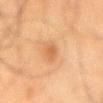Captured during whole-body skin photography for melanoma surveillance; the lesion was not biopsied. Automated tile analysis of the lesion measured a footprint of about 2.5 mm², a shape eccentricity near 0.95, and a shape-asymmetry score of about 0.4 (0 = symmetric). It also reported a classifier nevus-likeness of about 0/100 and a detector confidence of about 80 out of 100 that the crop contains a lesion. Approximately 3.5 mm at its widest. The tile uses cross-polarized illumination. A male subject, aged 63 to 67. A lesion tile, about 15 mm wide, cut from a 3D total-body photograph. The lesion is located on the arm.